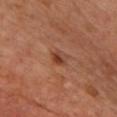<lesion>
  <biopsy_status>not biopsied; imaged during a skin examination</biopsy_status>
  <image>
    <source>total-body photography crop</source>
    <field_of_view_mm>15</field_of_view_mm>
  </image>
  <lighting>cross-polarized</lighting>
  <site>chest</site>
  <lesion_size>
    <long_diameter_mm_approx>2.0</long_diameter_mm_approx>
  </lesion_size>
  <patient>
    <age_approx>65</age_approx>
  </patient>
  <automated_metrics>
    <area_mm2_approx>3.0</area_mm2_approx>
    <eccentricity>0.4</eccentricity>
    <shape_asymmetry>0.2</shape_asymmetry>
  </automated_metrics>
</lesion>